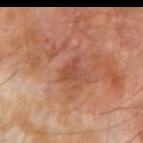workup — total-body-photography surveillance lesion; no biopsy
illumination — cross-polarized illumination
body site — the left forearm
acquisition — total-body-photography crop, ~15 mm field of view
size — ~3 mm (longest diameter)
patient — male, aged 68–72
automated lesion analysis — border irregularity of about 4 on a 0–10 scale, internal color variation of about 1 on a 0–10 scale, and peripheral color asymmetry of about 0.5; an automated nevus-likeness rating near 0 out of 100 and a lesion-detection confidence of about 100/100